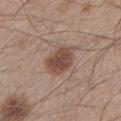Captured during whole-body skin photography for melanoma surveillance; the lesion was not biopsied.
The lesion is located on the leg.
A male patient, approximately 45 years of age.
A 15 mm crop from a total-body photograph taken for skin-cancer surveillance.